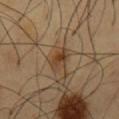Impression:
The lesion was tiled from a total-body skin photograph and was not biopsied.